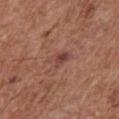Captured during whole-body skin photography for melanoma surveillance; the lesion was not biopsied.
A male subject approximately 75 years of age.
Longest diameter approximately 3 mm.
A 15 mm crop from a total-body photograph taken for skin-cancer surveillance.
Imaged with white-light lighting.
The lesion is located on the front of the torso.